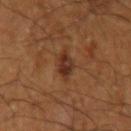Captured during whole-body skin photography for melanoma surveillance; the lesion was not biopsied. The subject is a male in their 60s. Located on the left upper arm. This is a cross-polarized tile. This image is a 15 mm lesion crop taken from a total-body photograph. The recorded lesion diameter is about 2.5 mm.